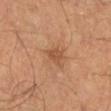  biopsy_status: not biopsied; imaged during a skin examination
  site: left lower leg
  lesion_size:
    long_diameter_mm_approx: 2.5
  image:
    source: total-body photography crop
    field_of_view_mm: 15
  lighting: cross-polarized
  patient:
    sex: male
    age_approx: 40